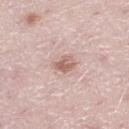No biopsy was performed on this lesion — it was imaged during a full skin examination and was not determined to be concerning.
About 2.5 mm across.
Cropped from a total-body skin-imaging series; the visible field is about 15 mm.
A male patient approximately 50 years of age.
This is a white-light tile.
The lesion is located on the left thigh.
The total-body-photography lesion software estimated a lesion area of about 4 mm², an eccentricity of roughly 0.65, and a shape-asymmetry score of about 0.25 (0 = symmetric). The software also gave a mean CIELAB color near L≈62 a*≈20 b*≈24 and roughly 11 lightness units darker than nearby skin.